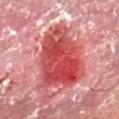biopsy status=catalogued during a skin exam; not biopsied
patient=male, in their mid-50s
lighting=cross-polarized illumination
acquisition=~15 mm crop, total-body skin-cancer survey
size=~9 mm (longest diameter)
anatomic site=the left thigh